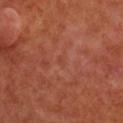Context:
The lesion is on the upper back. The lesion-visualizer software estimated a lesion area of about 1 mm², a shape eccentricity near 0.7, and a shape-asymmetry score of about 0.25 (0 = symmetric). And it measured border irregularity of about 2 on a 0–10 scale, internal color variation of about 0 on a 0–10 scale, and a peripheral color-asymmetry measure near 0. It also reported an automated nevus-likeness rating near 0 out of 100 and a lesion-detection confidence of about 100/100. This is a cross-polarized tile. A roughly 15 mm field-of-view crop from a total-body skin photograph. The patient is female. Measured at roughly 1 mm in maximum diameter.